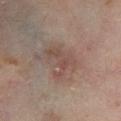On the right lower leg. The patient is a male aged approximately 70. A roughly 15 mm field-of-view crop from a total-body skin photograph. Automated tile analysis of the lesion measured a footprint of about 9 mm², an eccentricity of roughly 0.75, and a shape-asymmetry score of about 0.5 (0 = symmetric). Captured under cross-polarized illumination.